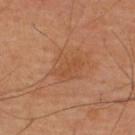Q: Was a biopsy performed?
A: imaged on a skin check; not biopsied
Q: What is the lesion's diameter?
A: about 4 mm
Q: What kind of image is this?
A: ~15 mm tile from a whole-body skin photo
Q: Where on the body is the lesion?
A: the upper back
Q: Illumination type?
A: cross-polarized
Q: Patient demographics?
A: male, aged 58–62